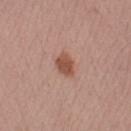Part of a total-body skin-imaging series; this lesion was reviewed on a skin check and was not flagged for biopsy.
Captured under white-light illumination.
Automated image analysis of the tile measured a footprint of about 5 mm², a shape eccentricity near 0.7, and a shape-asymmetry score of about 0.2 (0 = symmetric). It also reported a border-irregularity rating of about 2/10, a color-variation rating of about 2.5/10, and radial color variation of about 1. The analysis additionally found a lesion-detection confidence of about 100/100.
A male subject, approximately 55 years of age.
Cropped from a total-body skin-imaging series; the visible field is about 15 mm.
The lesion is located on the left upper arm.